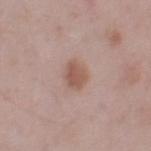Assessment:
Part of a total-body skin-imaging series; this lesion was reviewed on a skin check and was not flagged for biopsy.
Acquisition and patient details:
Captured under white-light illumination. A male subject, aged 73–77. From the leg. A 15 mm close-up extracted from a 3D total-body photography capture.